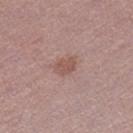notes=total-body-photography surveillance lesion; no biopsy
body site=the right thigh
subject=male, aged 58–62
imaging modality=~15 mm crop, total-body skin-cancer survey
lesion size=≈2.5 mm